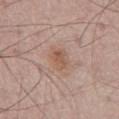Clinical impression:
The lesion was tiled from a total-body skin photograph and was not biopsied.
Clinical summary:
The lesion is on the back. A male patient, aged 63–67. The lesion's longest dimension is about 3 mm. Cropped from a whole-body photographic skin survey; the tile spans about 15 mm. Automated image analysis of the tile measured a lesion area of about 5 mm², an eccentricity of roughly 0.8, and a symmetry-axis asymmetry near 0.25.